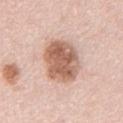<case>
<biopsy_status>not biopsied; imaged during a skin examination</biopsy_status>
<site>chest</site>
<lighting>white-light</lighting>
<lesion_size>
  <long_diameter_mm_approx>6.0</long_diameter_mm_approx>
</lesion_size>
<patient>
  <sex>male</sex>
  <age_approx>50</age_approx>
</patient>
<image>
  <source>total-body photography crop</source>
  <field_of_view_mm>15</field_of_view_mm>
</image>
</case>